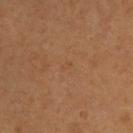Clinical impression:
Recorded during total-body skin imaging; not selected for excision or biopsy.
Background:
About 1.5 mm across. Cropped from a whole-body photographic skin survey; the tile spans about 15 mm. On the upper back. Captured under cross-polarized illumination. A male patient approximately 65 years of age.